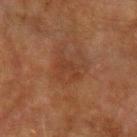This lesion was catalogued during total-body skin photography and was not selected for biopsy. A roughly 15 mm field-of-view crop from a total-body skin photograph. The lesion is located on the left upper arm. A male patient roughly 75 years of age.